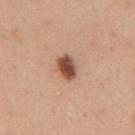Imaged during a routine full-body skin examination; the lesion was not biopsied and no histopathology is available.
A roughly 15 mm field-of-view crop from a total-body skin photograph.
Imaged with white-light lighting.
The total-body-photography lesion software estimated a mean CIELAB color near L≈50 a*≈23 b*≈29 and a normalized lesion–skin contrast near 11.5. And it measured a peripheral color-asymmetry measure near 2.
A male subject, aged 33 to 37.
On the mid back.
Approximately 3 mm at its widest.